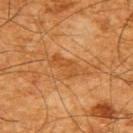Q: Was a biopsy performed?
A: total-body-photography surveillance lesion; no biopsy
Q: How was the tile lit?
A: cross-polarized illumination
Q: What are the patient's age and sex?
A: male, roughly 65 years of age
Q: How large is the lesion?
A: ~4.5 mm (longest diameter)
Q: What is the imaging modality?
A: 15 mm crop, total-body photography
Q: Lesion location?
A: the back